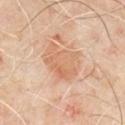Imaged during a routine full-body skin examination; the lesion was not biopsied and no histopathology is available. Automated image analysis of the tile measured an average lesion color of about L≈64 a*≈21 b*≈34 (CIELAB) and a lesion–skin lightness drop of about 8. The software also gave border irregularity of about 4 on a 0–10 scale, a color-variation rating of about 3.5/10, and peripheral color asymmetry of about 1. The subject is a male approximately 50 years of age. The lesion is on the front of the torso. Approximately 5 mm at its widest. A roughly 15 mm field-of-view crop from a total-body skin photograph. The tile uses cross-polarized illumination.